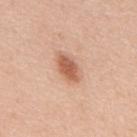No biopsy was performed on this lesion — it was imaged during a full skin examination and was not determined to be concerning. The lesion is on the upper back. About 4 mm across. Automated tile analysis of the lesion measured a nevus-likeness score of about 95/100 and a lesion-detection confidence of about 100/100. A 15 mm close-up extracted from a 3D total-body photography capture. Captured under white-light illumination. A male subject aged around 50.